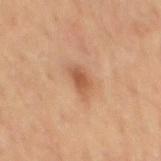Assessment:
The lesion was photographed on a routine skin check and not biopsied; there is no pathology result.
Clinical summary:
The tile uses cross-polarized illumination. The patient is a male in their mid- to late 50s. Approximately 4 mm at its widest. The total-body-photography lesion software estimated an outline eccentricity of about 0.85 (0 = round, 1 = elongated) and two-axis asymmetry of about 0.3. And it measured a lesion–skin lightness drop of about 10 and a normalized lesion–skin contrast near 7. The software also gave a classifier nevus-likeness of about 50/100 and lesion-presence confidence of about 100/100. A 15 mm close-up extracted from a 3D total-body photography capture. The lesion is located on the mid back.